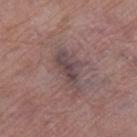biopsy_status: not biopsied; imaged during a skin examination
patient:
  sex: female
  age_approx: 70
site: leg
image:
  source: total-body photography crop
  field_of_view_mm: 15
automated_metrics:
  area_mm2_approx: 9.0
  cielab_L: 44
  cielab_a: 16
  cielab_b: 15
  vs_skin_darker_L: 8.0
  vs_skin_contrast_norm: 7.5
  border_irregularity_0_10: 4.5
  color_variation_0_10: 3.5
  peripheral_color_asymmetry: 1.5
lighting: white-light
lesion_size:
  long_diameter_mm_approx: 5.0